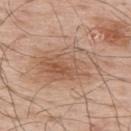This lesion was catalogued during total-body skin photography and was not selected for biopsy.
The subject is a male in their mid-60s.
Measured at roughly 7.5 mm in maximum diameter.
Cropped from a whole-body photographic skin survey; the tile spans about 15 mm.
Located on the upper back.